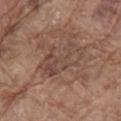{"biopsy_status": "not biopsied; imaged during a skin examination", "automated_metrics": {"nevus_likeness_0_100": 0, "lesion_detection_confidence_0_100": 95}, "site": "mid back", "lighting": "white-light", "image": {"source": "total-body photography crop", "field_of_view_mm": 15}, "patient": {"sex": "male", "age_approx": 80}}